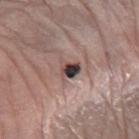Clinical impression:
The lesion was photographed on a routine skin check and not biopsied; there is no pathology result.
Acquisition and patient details:
A lesion tile, about 15 mm wide, cut from a 3D total-body photograph. The lesion is on the arm. The subject is a male roughly 65 years of age.